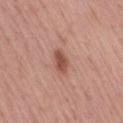No biopsy was performed on this lesion — it was imaged during a full skin examination and was not determined to be concerning.
Imaged with white-light lighting.
A 15 mm close-up tile from a total-body photography series done for melanoma screening.
The lesion is on the right thigh.
Automated tile analysis of the lesion measured an eccentricity of roughly 0.75 and a symmetry-axis asymmetry near 0.25. The software also gave a border-irregularity index near 2/10, a color-variation rating of about 1.5/10, and radial color variation of about 0.5.
A female patient approximately 55 years of age.